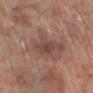Impression: This lesion was catalogued during total-body skin photography and was not selected for biopsy. Clinical summary: Cropped from a whole-body photographic skin survey; the tile spans about 15 mm. An algorithmic analysis of the crop reported a lesion color around L≈49 a*≈18 b*≈24 in CIELAB and a lesion-to-skin contrast of about 5 (normalized; higher = more distinct). The lesion is located on the left lower leg. A male subject, aged approximately 70. Longest diameter approximately 8.5 mm.